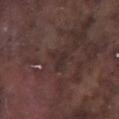The lesion was tiled from a total-body skin photograph and was not biopsied. A male patient, aged approximately 75. The lesion is located on the left lower leg. Captured under white-light illumination. Approximately 2.5 mm at its widest. A 15 mm close-up extracted from a 3D total-body photography capture.